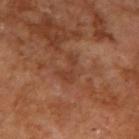{"biopsy_status": "not biopsied; imaged during a skin examination", "site": "arm", "patient": {"sex": "male", "age_approx": 55}, "image": {"source": "total-body photography crop", "field_of_view_mm": 15}, "automated_metrics": {"area_mm2_approx": 4.5, "eccentricity": 0.85, "shape_asymmetry": 0.5, "vs_skin_darker_L": 6.0, "vs_skin_contrast_norm": 5.5, "border_irregularity_0_10": 6.5, "peripheral_color_asymmetry": 0.5, "nevus_likeness_0_100": 0, "lesion_detection_confidence_0_100": 100}, "lighting": "cross-polarized"}